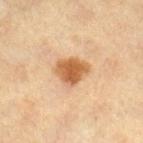biopsy status = total-body-photography surveillance lesion; no biopsy.